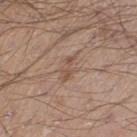An algorithmic analysis of the crop reported an area of roughly 3.5 mm², an eccentricity of roughly 0.85, and a shape-asymmetry score of about 0.35 (0 = symmetric). The software also gave an average lesion color of about L≈52 a*≈17 b*≈27 (CIELAB), a lesion–skin lightness drop of about 7, and a normalized lesion–skin contrast near 5.5. The software also gave a border-irregularity rating of about 4/10, internal color variation of about 1 on a 0–10 scale, and radial color variation of about 0. The software also gave lesion-presence confidence of about 100/100. The tile uses white-light illumination. A male subject, aged around 20. On the left thigh. A 15 mm close-up tile from a total-body photography series done for melanoma screening. About 3 mm across.